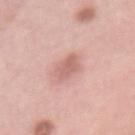{
  "biopsy_status": "not biopsied; imaged during a skin examination",
  "patient": {
    "sex": "female",
    "age_approx": 65
  },
  "lesion_size": {
    "long_diameter_mm_approx": 4.5
  },
  "automated_metrics": {
    "cielab_L": 63,
    "cielab_a": 24,
    "cielab_b": 25,
    "vs_skin_darker_L": 10.0,
    "vs_skin_contrast_norm": 6.5
  },
  "lighting": "white-light",
  "site": "mid back",
  "image": {
    "source": "total-body photography crop",
    "field_of_view_mm": 15
  }
}